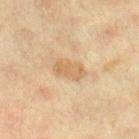Clinical impression:
No biopsy was performed on this lesion — it was imaged during a full skin examination and was not determined to be concerning.
Image and clinical context:
A 15 mm crop from a total-body photograph taken for skin-cancer surveillance. From the leg. A female subject about 40 years old. The tile uses cross-polarized illumination. Approximately 4 mm at its widest.